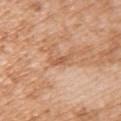Recorded during total-body skin imaging; not selected for excision or biopsy.
From the upper back.
Cropped from a total-body skin-imaging series; the visible field is about 15 mm.
A female patient, roughly 40 years of age.